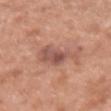Recorded during total-body skin imaging; not selected for excision or biopsy. On the right forearm. A female subject approximately 40 years of age. A region of skin cropped from a whole-body photographic capture, roughly 15 mm wide. Longest diameter approximately 6 mm.